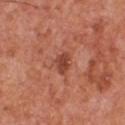<lesion>
<biopsy_status>not biopsied; imaged during a skin examination</biopsy_status>
<image>
  <source>total-body photography crop</source>
  <field_of_view_mm>15</field_of_view_mm>
</image>
<patient>
  <sex>male</sex>
  <age_approx>55</age_approx>
</patient>
<site>front of the torso</site>
</lesion>